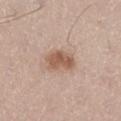workup: no biopsy performed (imaged during a skin exam)
body site: the left thigh
lesion diameter: about 4 mm
imaging modality: ~15 mm tile from a whole-body skin photo
automated lesion analysis: a mean CIELAB color near L≈56 a*≈19 b*≈29, roughly 12 lightness units darker than nearby skin, and a normalized border contrast of about 8.5; a border-irregularity rating of about 2.5/10
subject: male, approximately 65 years of age